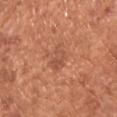biopsy status — total-body-photography surveillance lesion; no biopsy
lesion diameter — ≈3 mm
patient — male, about 65 years old
location — the chest
automated metrics — a footprint of about 5 mm²; a border-irregularity index near 3.5/10 and internal color variation of about 3.5 on a 0–10 scale; an automated nevus-likeness rating near 0 out of 100 and lesion-presence confidence of about 100/100
tile lighting — white-light
acquisition — ~15 mm tile from a whole-body skin photo